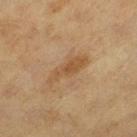Background: The lesion is located on the left thigh. A female patient in their 40s. Cropped from a total-body skin-imaging series; the visible field is about 15 mm.